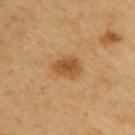Q: Is there a histopathology result?
A: imaged on a skin check; not biopsied
Q: Where on the body is the lesion?
A: the right upper arm
Q: Patient demographics?
A: male, approximately 60 years of age
Q: What is the imaging modality?
A: 15 mm crop, total-body photography